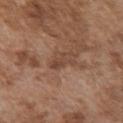Impression:
This lesion was catalogued during total-body skin photography and was not selected for biopsy.
Acquisition and patient details:
The lesion is located on the chest. A male patient, in their mid- to late 70s. Automated tile analysis of the lesion measured a lesion area of about 3.5 mm², an outline eccentricity of about 0.8 (0 = round, 1 = elongated), and a symmetry-axis asymmetry near 0.45. The analysis additionally found a normalized border contrast of about 6. It also reported border irregularity of about 5.5 on a 0–10 scale and internal color variation of about 1 on a 0–10 scale. Cropped from a total-body skin-imaging series; the visible field is about 15 mm.